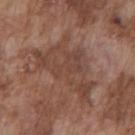Captured during whole-body skin photography for melanoma surveillance; the lesion was not biopsied. The tile uses white-light illumination. On the chest. The subject is a male aged 73 to 77. A region of skin cropped from a whole-body photographic capture, roughly 15 mm wide. Automated image analysis of the tile measured an average lesion color of about L≈43 a*≈19 b*≈26 (CIELAB), about 7 CIELAB-L* units darker than the surrounding skin, and a normalized border contrast of about 5.5. And it measured a detector confidence of about 50 out of 100 that the crop contains a lesion.